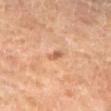Case summary:
- biopsy status · no biopsy performed (imaged during a skin exam)
- body site · the left lower leg
- tile lighting · cross-polarized illumination
- patient · female, aged approximately 70
- imaging modality · ~15 mm tile from a whole-body skin photo